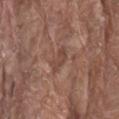notes — no biopsy performed (imaged during a skin exam)
lesion size — ≈3 mm
patient — male, in their 80s
illumination — white-light
body site — the lower back
imaging modality — total-body-photography crop, ~15 mm field of view